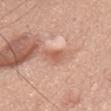follow-up: imaged on a skin check; not biopsied
size: about 2.5 mm
patient: male, aged approximately 75
site: the chest
TBP lesion metrics: a lesion area of about 3.5 mm², a shape eccentricity near 0.75, and two-axis asymmetry of about 0.45; a mean CIELAB color near L≈59 a*≈25 b*≈32 and a normalized lesion–skin contrast near 6
imaging modality: 15 mm crop, total-body photography